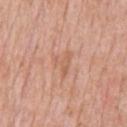Findings:
* subject — male, aged around 60
* illumination — white-light
* location — the chest
* size — ≈3.5 mm
* image — ~15 mm crop, total-body skin-cancer survey